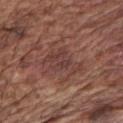The lesion was photographed on a routine skin check and not biopsied; there is no pathology result. Measured at roughly 4.5 mm in maximum diameter. Imaged with white-light lighting. The lesion is on the right upper arm. A 15 mm crop from a total-body photograph taken for skin-cancer surveillance. The subject is a male in their mid-70s.